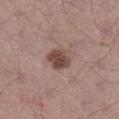The lesion was tiled from a total-body skin photograph and was not biopsied. From the leg. A male subject, aged 38–42. Cropped from a total-body skin-imaging series; the visible field is about 15 mm.